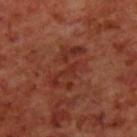Q: Was this lesion biopsied?
A: catalogued during a skin exam; not biopsied
Q: How was the tile lit?
A: cross-polarized illumination
Q: Where on the body is the lesion?
A: the upper back
Q: Patient demographics?
A: male, aged 68–72
Q: What is the imaging modality?
A: 15 mm crop, total-body photography